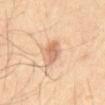Located on the abdomen.
Approximately 3 mm at its widest.
Cropped from a whole-body photographic skin survey; the tile spans about 15 mm.
The tile uses cross-polarized illumination.
A male patient, aged 53–57.